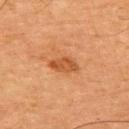  biopsy_status: not biopsied; imaged during a skin examination
  lesion_size:
    long_diameter_mm_approx: 3.5
  image:
    source: total-body photography crop
    field_of_view_mm: 15
  site: upper back
  lighting: cross-polarized
  patient:
    sex: male
    age_approx: 65